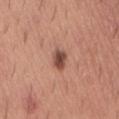Q: Is there a histopathology result?
A: no biopsy performed (imaged during a skin exam)
Q: What kind of image is this?
A: ~15 mm tile from a whole-body skin photo
Q: Automated lesion metrics?
A: a mean CIELAB color near L≈48 a*≈24 b*≈27, about 15 CIELAB-L* units darker than the surrounding skin, and a normalized lesion–skin contrast near 10; a border-irregularity index near 3/10 and a within-lesion color-variation index near 3.5/10
Q: Illumination type?
A: white-light
Q: Patient demographics?
A: male, in their 40s
Q: Lesion location?
A: the back